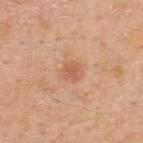Recorded during total-body skin imaging; not selected for excision or biopsy. Cropped from a whole-body photographic skin survey; the tile spans about 15 mm. A male subject aged 48 to 52. The tile uses white-light illumination. The recorded lesion diameter is about 2.5 mm. The lesion is on the upper back.